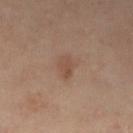{"biopsy_status": "not biopsied; imaged during a skin examination", "lighting": "cross-polarized", "image": {"source": "total-body photography crop", "field_of_view_mm": 15}, "patient": {"sex": "female", "age_approx": 40}, "site": "right leg", "lesion_size": {"long_diameter_mm_approx": 2.5}}